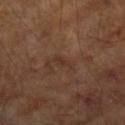Impression: The lesion was photographed on a routine skin check and not biopsied; there is no pathology result. Acquisition and patient details: A 15 mm close-up extracted from a 3D total-body photography capture. The subject is a male in their mid-60s. The lesion's longest dimension is about 2.5 mm. Located on the left forearm.